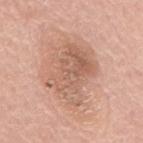<tbp_lesion>
  <biopsy_status>not biopsied; imaged during a skin examination</biopsy_status>
  <site>mid back</site>
  <automated_metrics>
    <cielab_L>60</cielab_L>
    <cielab_a>21</cielab_a>
    <cielab_b>29</cielab_b>
    <border_irregularity_0_10>4.0</border_irregularity_0_10>
    <color_variation_0_10>5.0</color_variation_0_10>
    <peripheral_color_asymmetry>2.0</peripheral_color_asymmetry>
    <lesion_detection_confidence_0_100>100</lesion_detection_confidence_0_100>
  </automated_metrics>
  <lesion_size>
    <long_diameter_mm_approx>7.0</long_diameter_mm_approx>
  </lesion_size>
  <patient>
    <sex>female</sex>
    <age_approx>60</age_approx>
  </patient>
  <image>
    <source>total-body photography crop</source>
    <field_of_view_mm>15</field_of_view_mm>
  </image>
</tbp_lesion>